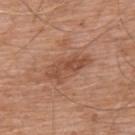{
  "biopsy_status": "not biopsied; imaged during a skin examination",
  "lesion_size": {
    "long_diameter_mm_approx": 5.5
  },
  "lighting": "white-light",
  "automated_metrics": {
    "border_irregularity_0_10": 3.0,
    "color_variation_0_10": 3.5,
    "peripheral_color_asymmetry": 1.0,
    "nevus_likeness_0_100": 5,
    "lesion_detection_confidence_0_100": 100
  },
  "site": "chest",
  "image": {
    "source": "total-body photography crop",
    "field_of_view_mm": 15
  },
  "patient": {
    "sex": "male",
    "age_approx": 60
  }
}